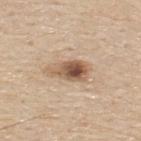Recorded during total-body skin imaging; not selected for excision or biopsy.
Automated image analysis of the tile measured a lesion area of about 10 mm², a shape eccentricity near 0.9, and two-axis asymmetry of about 0.25. The analysis additionally found a classifier nevus-likeness of about 95/100 and lesion-presence confidence of about 100/100.
The lesion is located on the upper back.
The tile uses white-light illumination.
A male patient, approximately 80 years of age.
Cropped from a whole-body photographic skin survey; the tile spans about 15 mm.
Approximately 5 mm at its widest.